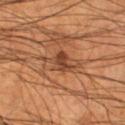follow-up: total-body-photography surveillance lesion; no biopsy
lesion size: ~4.5 mm (longest diameter)
automated metrics: an area of roughly 7.5 mm² and an outline eccentricity of about 0.85 (0 = round, 1 = elongated); a lesion color around L≈41 a*≈21 b*≈31 in CIELAB, a lesion–skin lightness drop of about 9, and a lesion-to-skin contrast of about 7.5 (normalized; higher = more distinct)
patient: male, approximately 55 years of age
anatomic site: the right lower leg
acquisition: 15 mm crop, total-body photography